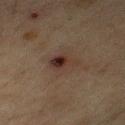Impression:
The lesion was photographed on a routine skin check and not biopsied; there is no pathology result.
Acquisition and patient details:
The patient is a male roughly 75 years of age. From the chest. A close-up tile cropped from a whole-body skin photograph, about 15 mm across.